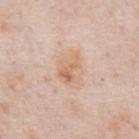<tbp_lesion>
  <biopsy_status>not biopsied; imaged during a skin examination</biopsy_status>
  <site>chest</site>
  <lesion_size>
    <long_diameter_mm_approx>3.5</long_diameter_mm_approx>
  </lesion_size>
  <patient>
    <sex>male</sex>
    <age_approx>80</age_approx>
  </patient>
  <image>
    <source>total-body photography crop</source>
    <field_of_view_mm>15</field_of_view_mm>
  </image>
  <lighting>white-light</lighting>
</tbp_lesion>